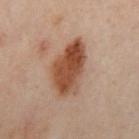Acquisition and patient details:
About 6.5 mm across. A 15 mm close-up extracted from a 3D total-body photography capture. Automated image analysis of the tile measured a footprint of about 19 mm² and a shape-asymmetry score of about 0.2 (0 = symmetric). And it measured border irregularity of about 2.5 on a 0–10 scale and a color-variation rating of about 6/10. The software also gave a classifier nevus-likeness of about 100/100 and lesion-presence confidence of about 100/100. Captured under cross-polarized illumination. From the right leg. A female subject, aged 58–62.